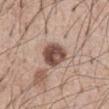<tbp_lesion>
  <biopsy_status>not biopsied; imaged during a skin examination</biopsy_status>
</tbp_lesion>